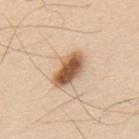Q: Is there a histopathology result?
A: catalogued during a skin exam; not biopsied
Q: What is the imaging modality?
A: ~15 mm crop, total-body skin-cancer survey
Q: What lighting was used for the tile?
A: white-light
Q: What is the anatomic site?
A: the upper back
Q: How large is the lesion?
A: ≈5 mm
Q: Automated lesion metrics?
A: a mean CIELAB color near L≈58 a*≈20 b*≈34
Q: Patient demographics?
A: male, aged 58–62